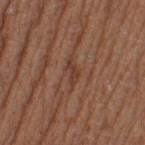{
  "biopsy_status": "not biopsied; imaged during a skin examination",
  "site": "left thigh",
  "lesion_size": {
    "long_diameter_mm_approx": 3.0
  },
  "lighting": "white-light",
  "image": {
    "source": "total-body photography crop",
    "field_of_view_mm": 15
  },
  "patient": {
    "sex": "female",
    "age_approx": 40
  }
}